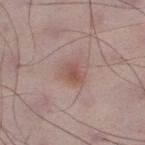{"biopsy_status": "not biopsied; imaged during a skin examination", "patient": {"sex": "male", "age_approx": 50}, "site": "left lower leg", "lesion_size": {"long_diameter_mm_approx": 3.0}, "image": {"source": "total-body photography crop", "field_of_view_mm": 15}, "lighting": "white-light", "automated_metrics": {"area_mm2_approx": 5.5, "eccentricity": 0.55, "shape_asymmetry": 0.3, "border_irregularity_0_10": 3.0, "color_variation_0_10": 3.5, "peripheral_color_asymmetry": 1.0}}